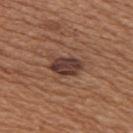Clinical impression:
Imaged during a routine full-body skin examination; the lesion was not biopsied and no histopathology is available.
Clinical summary:
Automated tile analysis of the lesion measured an area of roughly 7.5 mm² and two-axis asymmetry of about 0.25. The analysis additionally found a lesion color around L≈38 a*≈19 b*≈24 in CIELAB and roughly 12 lightness units darker than nearby skin. And it measured a nevus-likeness score of about 45/100 and a detector confidence of about 100 out of 100 that the crop contains a lesion. Imaged with white-light lighting. The recorded lesion diameter is about 4 mm. Cropped from a total-body skin-imaging series; the visible field is about 15 mm. A female patient, in their mid- to late 50s. Located on the right upper arm.